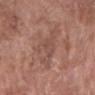This lesion was catalogued during total-body skin photography and was not selected for biopsy. The total-body-photography lesion software estimated an area of roughly 12 mm². The software also gave border irregularity of about 8 on a 0–10 scale, a within-lesion color-variation index near 2/10, and radial color variation of about 0.5. The software also gave a classifier nevus-likeness of about 0/100 and a detector confidence of about 70 out of 100 that the crop contains a lesion. The recorded lesion diameter is about 6.5 mm. A 15 mm close-up tile from a total-body photography series done for melanoma screening. On the arm. The patient is a female approximately 70 years of age. This is a white-light tile.